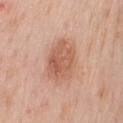Part of a total-body skin-imaging series; this lesion was reviewed on a skin check and was not flagged for biopsy. Longest diameter approximately 5.5 mm. The total-body-photography lesion software estimated a lesion–skin lightness drop of about 10. The software also gave a border-irregularity rating of about 2/10 and a within-lesion color-variation index near 4/10. The software also gave a nevus-likeness score of about 45/100 and a detector confidence of about 100 out of 100 that the crop contains a lesion. A female patient aged approximately 50. Located on the mid back. A lesion tile, about 15 mm wide, cut from a 3D total-body photograph. The tile uses white-light illumination.